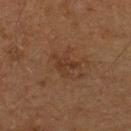No biopsy was performed on this lesion — it was imaged during a full skin examination and was not determined to be concerning.
A male subject approximately 65 years of age.
Automated image analysis of the tile measured a lesion color around L≈28 a*≈16 b*≈25 in CIELAB, about 5 CIELAB-L* units darker than the surrounding skin, and a lesion-to-skin contrast of about 5.5 (normalized; higher = more distinct). The software also gave a border-irregularity rating of about 5.5/10, a color-variation rating of about 2.5/10, and peripheral color asymmetry of about 1. The software also gave an automated nevus-likeness rating near 0 out of 100 and a lesion-detection confidence of about 100/100.
A roughly 15 mm field-of-view crop from a total-body skin photograph.
Located on the upper back.
The lesion's longest dimension is about 3 mm.